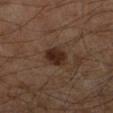Clinical impression:
The lesion was photographed on a routine skin check and not biopsied; there is no pathology result.
Clinical summary:
The lesion is on the left lower leg. A male patient aged 58 to 62. This is a cross-polarized tile. A 15 mm crop from a total-body photograph taken for skin-cancer surveillance.